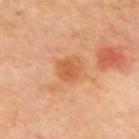biopsy status=imaged on a skin check; not biopsied
lesion size=about 3 mm
tile lighting=cross-polarized
site=the upper back
imaging modality=~15 mm crop, total-body skin-cancer survey